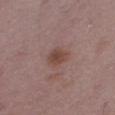Imaged during a routine full-body skin examination; the lesion was not biopsied and no histopathology is available. A 15 mm close-up extracted from a 3D total-body photography capture. Automated tile analysis of the lesion measured a footprint of about 5 mm², an outline eccentricity of about 0.55 (0 = round, 1 = elongated), and a symmetry-axis asymmetry near 0.2. The analysis additionally found a mean CIELAB color near L≈44 a*≈19 b*≈23, about 8 CIELAB-L* units darker than the surrounding skin, and a normalized border contrast of about 7.5. From the right thigh. The patient is a female aged approximately 50.